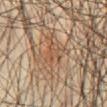Clinical impression: Recorded during total-body skin imaging; not selected for excision or biopsy. Acquisition and patient details: Cropped from a whole-body photographic skin survey; the tile spans about 15 mm. The patient is a male roughly 65 years of age. The lesion is on the front of the torso.